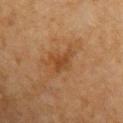biopsy status = total-body-photography surveillance lesion; no biopsy | image source = total-body-photography crop, ~15 mm field of view | subject = female, aged around 55 | diameter = about 3.5 mm | image-analysis metrics = a classifier nevus-likeness of about 0/100 and lesion-presence confidence of about 100/100 | illumination = cross-polarized | location = the right upper arm.